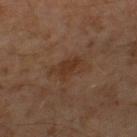Q: Was a biopsy performed?
A: no biopsy performed (imaged during a skin exam)
Q: Who is the patient?
A: male, aged 58–62
Q: Lesion location?
A: the right thigh
Q: How was this image acquired?
A: total-body-photography crop, ~15 mm field of view